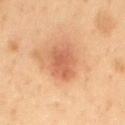The lesion's longest dimension is about 4 mm.
Automated image analysis of the tile measured an average lesion color of about L≈49 a*≈22 b*≈30 (CIELAB), about 8 CIELAB-L* units darker than the surrounding skin, and a lesion-to-skin contrast of about 6.5 (normalized; higher = more distinct). It also reported a within-lesion color-variation index near 2.5/10 and peripheral color asymmetry of about 1. The software also gave a lesion-detection confidence of about 100/100.
This is a cross-polarized tile.
A roughly 15 mm field-of-view crop from a total-body skin photograph.
From the mid back.
A male subject aged approximately 55.